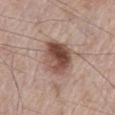workup = no biopsy performed (imaged during a skin exam)
tile lighting = white-light
location = the left thigh
imaging modality = ~15 mm tile from a whole-body skin photo
patient = male, aged 68 to 72
size = ~4.5 mm (longest diameter)